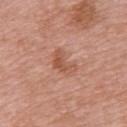<lesion>
<image>
  <source>total-body photography crop</source>
  <field_of_view_mm>15</field_of_view_mm>
</image>
<patient>
  <sex>female</sex>
  <age_approx>70</age_approx>
</patient>
<lighting>white-light</lighting>
<lesion_size>
  <long_diameter_mm_approx>3.5</long_diameter_mm_approx>
</lesion_size>
<site>upper back</site>
</lesion>